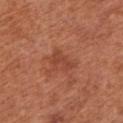The lesion was photographed on a routine skin check and not biopsied; there is no pathology result. The lesion is on the left arm. A close-up tile cropped from a whole-body skin photograph, about 15 mm across. Approximately 3 mm at its widest. A female patient, about 65 years old. Captured under white-light illumination.